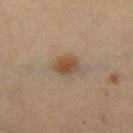<record>
<biopsy_status>not biopsied; imaged during a skin examination</biopsy_status>
<patient>
  <sex>female</sex>
  <age_approx>55</age_approx>
</patient>
<site>leg</site>
<lighting>cross-polarized</lighting>
<image>
  <source>total-body photography crop</source>
  <field_of_view_mm>15</field_of_view_mm>
</image>
</record>